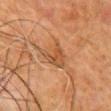The lesion was tiled from a total-body skin photograph and was not biopsied. From the chest. Longest diameter approximately 4 mm. A close-up tile cropped from a whole-body skin photograph, about 15 mm across. A male patient aged around 80. The lesion-visualizer software estimated a footprint of about 8.5 mm², an eccentricity of roughly 0.7, and a shape-asymmetry score of about 0.4 (0 = symmetric). It also reported a color-variation rating of about 4/10 and radial color variation of about 1.5. The analysis additionally found an automated nevus-likeness rating near 0 out of 100 and a lesion-detection confidence of about 95/100.